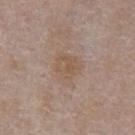follow-up: catalogued during a skin exam; not biopsied
subject: male, aged around 55
anatomic site: the front of the torso
image source: total-body-photography crop, ~15 mm field of view
lighting: white-light
TBP lesion metrics: a footprint of about 9.5 mm², an outline eccentricity of about 0.5 (0 = round, 1 = elongated), and a symmetry-axis asymmetry near 0.35; an average lesion color of about L≈54 a*≈15 b*≈27 (CIELAB), about 6 CIELAB-L* units darker than the surrounding skin, and a normalized border contrast of about 5.5
lesion diameter: about 4 mm